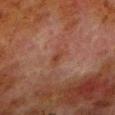Impression:
Recorded during total-body skin imaging; not selected for excision or biopsy.